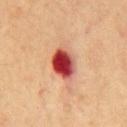biopsy status=catalogued during a skin exam; not biopsied | site=the chest | diameter=~4 mm (longest diameter) | image=15 mm crop, total-body photography | subject=male, roughly 70 years of age | illumination=cross-polarized.